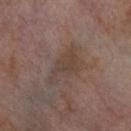Recorded during total-body skin imaging; not selected for excision or biopsy. This is a cross-polarized tile. A 15 mm close-up extracted from a 3D total-body photography capture. Measured at roughly 5.5 mm in maximum diameter. A female patient, aged 53–57. Located on the right thigh. The total-body-photography lesion software estimated a lesion area of about 9.5 mm², a shape eccentricity near 0.9, and two-axis asymmetry of about 0.45. It also reported a lesion–skin lightness drop of about 6 and a normalized lesion–skin contrast near 6. And it measured a nevus-likeness score of about 0/100.